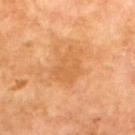automated lesion analysis=an average lesion color of about L≈61 a*≈25 b*≈44 (CIELAB), a lesion–skin lightness drop of about 7, and a normalized lesion–skin contrast near 4.5; a classifier nevus-likeness of about 0/100 and a lesion-detection confidence of about 100/100 | subject=male, aged approximately 70 | lighting=cross-polarized | location=the upper back | image=15 mm crop, total-body photography | lesion diameter=about 5 mm.